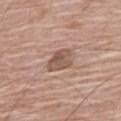<lesion>
  <biopsy_status>not biopsied; imaged during a skin examination</biopsy_status>
  <image>
    <source>total-body photography crop</source>
    <field_of_view_mm>15</field_of_view_mm>
  </image>
  <lighting>white-light</lighting>
  <site>right thigh</site>
  <patient>
    <sex>female</sex>
    <age_approx>75</age_approx>
  </patient>
</lesion>